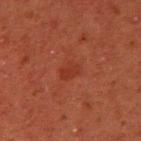The lesion-visualizer software estimated a footprint of about 4 mm², an outline eccentricity of about 0.65 (0 = round, 1 = elongated), and two-axis asymmetry of about 0.25. It also reported a border-irregularity index near 2.5/10, a color-variation rating of about 1.5/10, and a peripheral color-asymmetry measure near 0.5. The analysis additionally found a classifier nevus-likeness of about 5/100 and a detector confidence of about 100 out of 100 that the crop contains a lesion.
The subject is a male aged 43 to 47.
The tile uses cross-polarized illumination.
Approximately 2.5 mm at its widest.
Located on the right upper arm.
Cropped from a whole-body photographic skin survey; the tile spans about 15 mm.